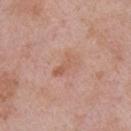Assessment: Part of a total-body skin-imaging series; this lesion was reviewed on a skin check and was not flagged for biopsy. Context: Located on the right upper arm. The tile uses white-light illumination. Automated tile analysis of the lesion measured an area of roughly 4.5 mm², a shape eccentricity near 0.85, and a shape-asymmetry score of about 0.3 (0 = symmetric). The analysis additionally found an automated nevus-likeness rating near 0 out of 100 and a lesion-detection confidence of about 100/100. A male patient in their mid-50s. Measured at roughly 3 mm in maximum diameter. This image is a 15 mm lesion crop taken from a total-body photograph.